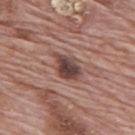The lesion was photographed on a routine skin check and not biopsied; there is no pathology result.